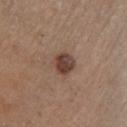No biopsy was performed on this lesion — it was imaged during a full skin examination and was not determined to be concerning.